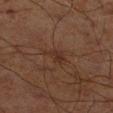Q: Was a biopsy performed?
A: catalogued during a skin exam; not biopsied
Q: Lesion size?
A: about 3.5 mm
Q: Illumination type?
A: cross-polarized
Q: How was this image acquired?
A: total-body-photography crop, ~15 mm field of view
Q: Who is the patient?
A: male, aged approximately 70
Q: Automated lesion metrics?
A: a lesion area of about 4 mm², an eccentricity of roughly 0.85, and a symmetry-axis asymmetry near 0.45; a border-irregularity index near 5/10, a within-lesion color-variation index near 1.5/10, and radial color variation of about 0.5; a nevus-likeness score of about 0/100
Q: Lesion location?
A: the right lower leg